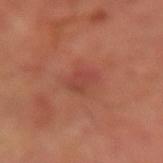The lesion was tiled from a total-body skin photograph and was not biopsied.